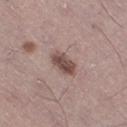biopsy status: total-body-photography surveillance lesion; no biopsy
subject: male, roughly 45 years of age
image source: 15 mm crop, total-body photography
tile lighting: white-light
body site: the leg